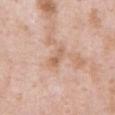Clinical impression: The lesion was tiled from a total-body skin photograph and was not biopsied. Acquisition and patient details: An algorithmic analysis of the crop reported an eccentricity of roughly 0.95 and two-axis asymmetry of about 0.4. The analysis additionally found a mean CIELAB color near L≈62 a*≈20 b*≈32, about 8 CIELAB-L* units darker than the surrounding skin, and a normalized lesion–skin contrast near 6. The software also gave a nevus-likeness score of about 0/100. A male subject, roughly 55 years of age. From the arm. A lesion tile, about 15 mm wide, cut from a 3D total-body photograph.